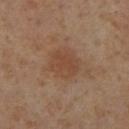{"biopsy_status": "not biopsied; imaged during a skin examination", "lighting": "cross-polarized", "lesion_size": {"long_diameter_mm_approx": 3.5}, "automated_metrics": {"area_mm2_approx": 10.0, "eccentricity": 0.45, "shape_asymmetry": 0.15, "vs_skin_darker_L": 6.0, "vs_skin_contrast_norm": 6.0}, "site": "right lower leg", "image": {"source": "total-body photography crop", "field_of_view_mm": 15}, "patient": {"sex": "female", "age_approx": 55}}